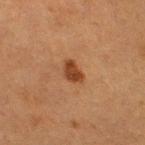biopsy status: imaged on a skin check; not biopsied | lighting: cross-polarized | subject: female, approximately 50 years of age | image source: total-body-photography crop, ~15 mm field of view | body site: the right thigh.